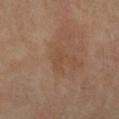{
  "biopsy_status": "not biopsied; imaged during a skin examination",
  "lighting": "cross-polarized",
  "patient": {
    "sex": "male",
    "age_approx": 50
  },
  "image": {
    "source": "total-body photography crop",
    "field_of_view_mm": 15
  },
  "lesion_size": {
    "long_diameter_mm_approx": 2.5
  },
  "site": "left forearm"
}